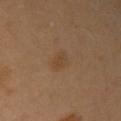Assessment: This lesion was catalogued during total-body skin photography and was not selected for biopsy. Background: From the right upper arm. The patient is a male aged 38–42. The total-body-photography lesion software estimated an average lesion color of about L≈43 a*≈16 b*≈32 (CIELAB) and about 5 CIELAB-L* units darker than the surrounding skin. The software also gave internal color variation of about 2 on a 0–10 scale and peripheral color asymmetry of about 1. It also reported an automated nevus-likeness rating near 10 out of 100 and a detector confidence of about 100 out of 100 that the crop contains a lesion. This image is a 15 mm lesion crop taken from a total-body photograph. Captured under cross-polarized illumination. Longest diameter approximately 2.5 mm.